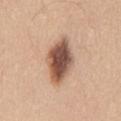| key | value |
|---|---|
| follow-up | no biopsy performed (imaged during a skin exam) |
| acquisition | 15 mm crop, total-body photography |
| subject | male, aged approximately 40 |
| lesion size | ≈6.5 mm |
| lighting | white-light |
| TBP lesion metrics | an area of roughly 16 mm² and a shape eccentricity near 0.85; a lesion color around L≈54 a*≈20 b*≈29 in CIELAB and a lesion–skin lightness drop of about 18; a border-irregularity index near 2/10, a within-lesion color-variation index near 7/10, and peripheral color asymmetry of about 2; a nevus-likeness score of about 95/100 |
| site | the mid back |